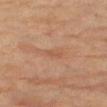Impression: Imaged during a routine full-body skin examination; the lesion was not biopsied and no histopathology is available. Clinical summary: The lesion is on the right thigh. Approximately 3 mm at its widest. Imaged with cross-polarized lighting. A 15 mm crop from a total-body photograph taken for skin-cancer surveillance. A female subject, roughly 80 years of age.